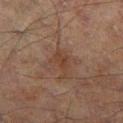Impression:
No biopsy was performed on this lesion — it was imaged during a full skin examination and was not determined to be concerning.
Acquisition and patient details:
A close-up tile cropped from a whole-body skin photograph, about 15 mm across. The patient is a male aged around 70. Located on the left thigh. Captured under cross-polarized illumination.